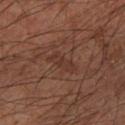follow-up — imaged on a skin check; not biopsied | anatomic site — the left forearm | size — ~3.5 mm (longest diameter) | acquisition — total-body-photography crop, ~15 mm field of view | tile lighting — cross-polarized | automated metrics — a footprint of about 4.5 mm²; a classifier nevus-likeness of about 0/100 and lesion-presence confidence of about 90/100 | subject — male, aged 63–67.